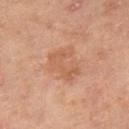{"automated_metrics": {"nevus_likeness_0_100": 0, "lesion_detection_confidence_0_100": 100}, "image": {"source": "total-body photography crop", "field_of_view_mm": 15}, "lighting": "cross-polarized", "site": "right thigh", "patient": {"sex": "male", "age_approx": 60}}